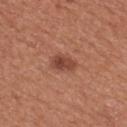biopsy_status: not biopsied; imaged during a skin examination
lesion_size:
  long_diameter_mm_approx: 3.0
patient:
  sex: male
  age_approx: 65
site: mid back
image:
  source: total-body photography crop
  field_of_view_mm: 15
lighting: white-light